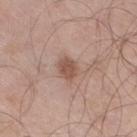biopsy status = imaged on a skin check; not biopsied | size = ≈2.5 mm | illumination = white-light illumination | patient = male, roughly 70 years of age | image = ~15 mm tile from a whole-body skin photo | anatomic site = the leg.